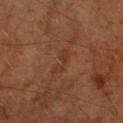No biopsy was performed on this lesion — it was imaged during a full skin examination and was not determined to be concerning.
A close-up tile cropped from a whole-body skin photograph, about 15 mm across.
The tile uses cross-polarized illumination.
Located on the right upper arm.
Approximately 4 mm at its widest.
The lesion-visualizer software estimated a lesion color around L≈28 a*≈18 b*≈26 in CIELAB, a lesion–skin lightness drop of about 4, and a normalized lesion–skin contrast near 5.
A male subject aged around 70.